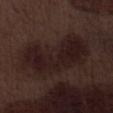Clinical impression: The lesion was tiled from a total-body skin photograph and was not biopsied. Image and clinical context: A male patient aged approximately 70. This is a white-light tile. A roughly 15 mm field-of-view crop from a total-body skin photograph. The total-body-photography lesion software estimated a lesion color around L≈18 a*≈14 b*≈15 in CIELAB and roughly 6 lightness units darker than nearby skin. And it measured a within-lesion color-variation index near 3.5/10 and peripheral color asymmetry of about 1. And it measured a classifier nevus-likeness of about 0/100 and a detector confidence of about 100 out of 100 that the crop contains a lesion. Longest diameter approximately 9 mm. From the right lower leg.